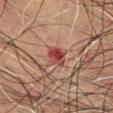biopsy_status: not biopsied; imaged during a skin examination
image:
  source: total-body photography crop
  field_of_view_mm: 15
site: chest
lighting: cross-polarized
patient:
  sex: male
  age_approx: 75
lesion_size:
  long_diameter_mm_approx: 2.5